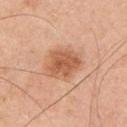{
  "biopsy_status": "not biopsied; imaged during a skin examination"
}